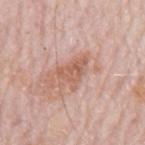{"biopsy_status": "not biopsied; imaged during a skin examination", "image": {"source": "total-body photography crop", "field_of_view_mm": 15}, "patient": {"sex": "male", "age_approx": 80}, "site": "mid back"}